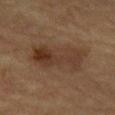This lesion was catalogued during total-body skin photography and was not selected for biopsy. About 7 mm across. This is a cross-polarized tile. From the abdomen. A region of skin cropped from a whole-body photographic capture, roughly 15 mm wide. A male patient about 85 years old or older.